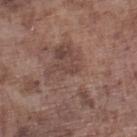Imaged during a routine full-body skin examination; the lesion was not biopsied and no histopathology is available.
The lesion is located on the left lower leg.
The recorded lesion diameter is about 2.5 mm.
Imaged with white-light lighting.
The subject is a male in their mid- to late 70s.
Automated tile analysis of the lesion measured a mean CIELAB color near L≈43 a*≈18 b*≈23 and a lesion–skin lightness drop of about 6. It also reported a border-irregularity index near 5/10, a color-variation rating of about 0/10, and a peripheral color-asymmetry measure near 0. The analysis additionally found a nevus-likeness score of about 0/100 and a detector confidence of about 80 out of 100 that the crop contains a lesion.
A region of skin cropped from a whole-body photographic capture, roughly 15 mm wide.